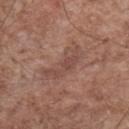follow-up: catalogued during a skin exam; not biopsied | tile lighting: white-light illumination | diameter: about 5.5 mm | patient: male, aged 68–72 | imaging modality: total-body-photography crop, ~15 mm field of view | automated metrics: an area of roughly 9.5 mm² and a shape eccentricity near 0.95; about 7 CIELAB-L* units darker than the surrounding skin and a normalized border contrast of about 5.5; a classifier nevus-likeness of about 0/100 and a detector confidence of about 80 out of 100 that the crop contains a lesion | anatomic site: the chest.